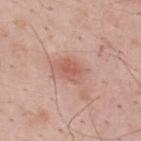Q: Was this lesion biopsied?
A: no biopsy performed (imaged during a skin exam)
Q: Lesion location?
A: the back
Q: What did automated image analysis measure?
A: a lesion color around L≈58 a*≈25 b*≈29 in CIELAB, a lesion–skin lightness drop of about 9, and a normalized border contrast of about 6; a border-irregularity rating of about 3/10, a color-variation rating of about 3.5/10, and radial color variation of about 1
Q: Lesion size?
A: ≈3 mm
Q: How was this image acquired?
A: 15 mm crop, total-body photography
Q: Who is the patient?
A: male, about 35 years old
Q: How was the tile lit?
A: white-light illumination